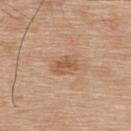| key | value |
|---|---|
| biopsy status | no biopsy performed (imaged during a skin exam) |
| image | ~15 mm tile from a whole-body skin photo |
| illumination | white-light |
| site | the upper back |
| automated lesion analysis | a footprint of about 5 mm², an outline eccentricity of about 0.8 (0 = round, 1 = elongated), and two-axis asymmetry of about 0.3; a color-variation rating of about 2.5/10 |
| lesion size | ~3 mm (longest diameter) |
| patient | male, aged around 75 |